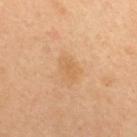{
  "biopsy_status": "not biopsied; imaged during a skin examination",
  "image": {
    "source": "total-body photography crop",
    "field_of_view_mm": 15
  },
  "lighting": "cross-polarized",
  "lesion_size": {
    "long_diameter_mm_approx": 3.0
  },
  "site": "upper back",
  "patient": {
    "sex": "female",
    "age_approx": 35
  }
}